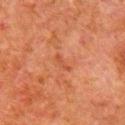follow-up — no biopsy performed (imaged during a skin exam)
imaging modality — 15 mm crop, total-body photography
anatomic site — the arm
lighting — cross-polarized
patient — male, aged approximately 80
image-analysis metrics — a lesion area of about 2 mm², an eccentricity of roughly 0.95, and two-axis asymmetry of about 0.35; a lesion color around L≈41 a*≈26 b*≈33 in CIELAB, about 5 CIELAB-L* units darker than the surrounding skin, and a lesion-to-skin contrast of about 5 (normalized; higher = more distinct); a border-irregularity index near 3.5/10, a within-lesion color-variation index near 0/10, and radial color variation of about 0; a nevus-likeness score of about 0/100 and a detector confidence of about 100 out of 100 that the crop contains a lesion
lesion diameter — ≈2.5 mm